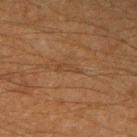Impression: No biopsy was performed on this lesion — it was imaged during a full skin examination and was not determined to be concerning.